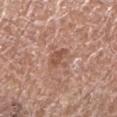Q: Is there a histopathology result?
A: no biopsy performed (imaged during a skin exam)
Q: How was this image acquired?
A: ~15 mm tile from a whole-body skin photo
Q: How large is the lesion?
A: ~3 mm (longest diameter)
Q: Who is the patient?
A: female, approximately 75 years of age
Q: Lesion location?
A: the left lower leg
Q: What did automated image analysis measure?
A: a footprint of about 4.5 mm² and an outline eccentricity of about 0.75 (0 = round, 1 = elongated); an average lesion color of about L≈52 a*≈23 b*≈28 (CIELAB), a lesion–skin lightness drop of about 9, and a normalized border contrast of about 6.5; border irregularity of about 5 on a 0–10 scale, internal color variation of about 1.5 on a 0–10 scale, and radial color variation of about 0.5; a classifier nevus-likeness of about 0/100
Q: What lighting was used for the tile?
A: white-light illumination